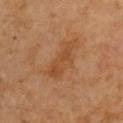notes — no biopsy performed (imaged during a skin exam)
image source — ~15 mm tile from a whole-body skin photo
location — the left arm
lesion size — about 5 mm
subject — male, aged 63 to 67